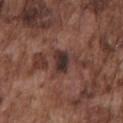Assessment:
This lesion was catalogued during total-body skin photography and was not selected for biopsy.
Background:
A 15 mm crop from a total-body photograph taken for skin-cancer surveillance. Automated tile analysis of the lesion measured a footprint of about 5 mm², a shape eccentricity near 0.8, and two-axis asymmetry of about 0.3. The software also gave a lesion–skin lightness drop of about 12 and a lesion-to-skin contrast of about 12.5 (normalized; higher = more distinct). The software also gave border irregularity of about 2.5 on a 0–10 scale, internal color variation of about 4.5 on a 0–10 scale, and a peripheral color-asymmetry measure near 1.5. The software also gave a classifier nevus-likeness of about 10/100. This is a white-light tile. The lesion is located on the front of the torso. Approximately 3 mm at its widest. The patient is a male about 75 years old.